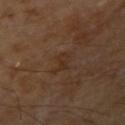The lesion was photographed on a routine skin check and not biopsied; there is no pathology result.